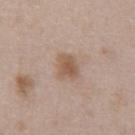Assessment: Part of a total-body skin-imaging series; this lesion was reviewed on a skin check and was not flagged for biopsy. Background: A lesion tile, about 15 mm wide, cut from a 3D total-body photograph. Approximately 3 mm at its widest. The lesion is located on the abdomen. A male subject, in their 50s.